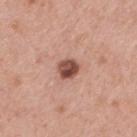The lesion was photographed on a routine skin check and not biopsied; there is no pathology result. The patient is a male roughly 40 years of age. A lesion tile, about 15 mm wide, cut from a 3D total-body photograph. Imaged with white-light lighting. Measured at roughly 2.5 mm in maximum diameter. From the mid back.